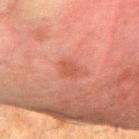This lesion was catalogued during total-body skin photography and was not selected for biopsy.
A roughly 15 mm field-of-view crop from a total-body skin photograph.
From the right forearm.
A male subject approximately 80 years of age.